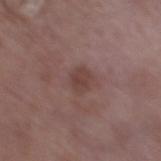* biopsy status: catalogued during a skin exam; not biopsied
* acquisition: total-body-photography crop, ~15 mm field of view
* subject: female, in their 70s
* site: the right thigh